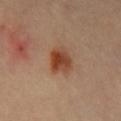{"biopsy_status": "not biopsied; imaged during a skin examination", "image": {"source": "total-body photography crop", "field_of_view_mm": 15}, "site": "chest", "automated_metrics": {"border_irregularity_0_10": 2.0, "color_variation_0_10": 6.5, "peripheral_color_asymmetry": 2.0}, "lesion_size": {"long_diameter_mm_approx": 4.0}}